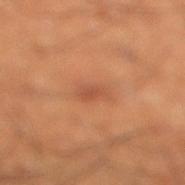The lesion was photographed on a routine skin check and not biopsied; there is no pathology result. A lesion tile, about 15 mm wide, cut from a 3D total-body photograph. Located on the leg. A male subject, aged approximately 50. About 3 mm across.